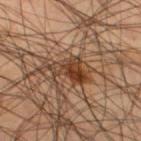Q: Is there a histopathology result?
A: catalogued during a skin exam; not biopsied
Q: Who is the patient?
A: male, roughly 45 years of age
Q: Where on the body is the lesion?
A: the right thigh
Q: What kind of image is this?
A: 15 mm crop, total-body photography
Q: Automated lesion metrics?
A: a footprint of about 8.5 mm², a shape eccentricity near 0.8, and a shape-asymmetry score of about 0.55 (0 = symmetric); about 11 CIELAB-L* units darker than the surrounding skin and a normalized lesion–skin contrast near 9.5; a border-irregularity index near 6/10, a within-lesion color-variation index near 8/10, and a peripheral color-asymmetry measure near 3; an automated nevus-likeness rating near 95 out of 100 and a detector confidence of about 100 out of 100 that the crop contains a lesion